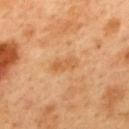Impression: The lesion was tiled from a total-body skin photograph and was not biopsied. Background: The lesion's longest dimension is about 3.5 mm. On the mid back. Imaged with cross-polarized lighting. A 15 mm crop from a total-body photograph taken for skin-cancer surveillance. The subject is a male approximately 50 years of age.